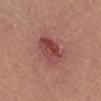site: right lower leg
image:
  source: total-body photography crop
  field_of_view_mm: 15
patient:
  sex: female
  age_approx: 45
lesion_size:
  long_diameter_mm_approx: 4.5